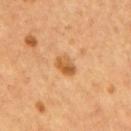Captured during whole-body skin photography for melanoma surveillance; the lesion was not biopsied.
A 15 mm crop from a total-body photograph taken for skin-cancer surveillance.
A male patient, in their mid-50s.
Automated tile analysis of the lesion measured a lesion color around L≈53 a*≈23 b*≈40 in CIELAB and a normalized border contrast of about 8. The analysis additionally found a border-irregularity rating of about 2/10, a within-lesion color-variation index near 5.5/10, and radial color variation of about 2. The analysis additionally found an automated nevus-likeness rating near 80 out of 100.
This is a cross-polarized tile.
The lesion is on the back.
The recorded lesion diameter is about 3 mm.